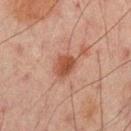workup = imaged on a skin check; not biopsied | location = the mid back | lesion size = ~3 mm (longest diameter) | image source = ~15 mm crop, total-body skin-cancer survey | lighting = cross-polarized illumination | subject = male, aged approximately 65.